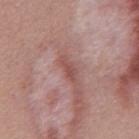Assessment: No biopsy was performed on this lesion — it was imaged during a full skin examination and was not determined to be concerning. Background: The total-body-photography lesion software estimated a footprint of about 3 mm², an outline eccentricity of about 0.9 (0 = round, 1 = elongated), and a symmetry-axis asymmetry near 0.45. The software also gave a lesion color around L≈50 a*≈25 b*≈23 in CIELAB and a lesion-to-skin contrast of about 6.5 (normalized; higher = more distinct). The analysis additionally found a lesion-detection confidence of about 95/100. A roughly 15 mm field-of-view crop from a total-body skin photograph. The patient is a male roughly 55 years of age. This is a white-light tile. Located on the mid back.